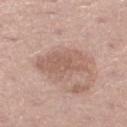Q: How was the tile lit?
A: white-light
Q: Lesion location?
A: the leg
Q: What is the imaging modality?
A: ~15 mm crop, total-body skin-cancer survey
Q: How large is the lesion?
A: ~5.5 mm (longest diameter)
Q: What are the patient's age and sex?
A: male, in their mid- to late 50s